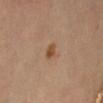Background: An algorithmic analysis of the crop reported a footprint of about 3 mm², an outline eccentricity of about 0.8 (0 = round, 1 = elongated), and a shape-asymmetry score of about 0.2 (0 = symmetric). About 2.5 mm across. A close-up tile cropped from a whole-body skin photograph, about 15 mm across. On the abdomen. The subject is a female aged around 65. Captured under cross-polarized illumination.